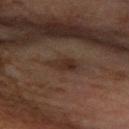biopsy status: catalogued during a skin exam; not biopsied
subject: female, about 60 years old
image: ~15 mm tile from a whole-body skin photo
diameter: ≈3.5 mm
lighting: cross-polarized
site: the left forearm